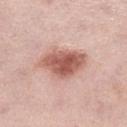Findings:
– TBP lesion metrics — a border-irregularity rating of about 2.5/10 and radial color variation of about 1.5
– lesion diameter — about 5.5 mm
– subject — female, aged around 65
– image — ~15 mm tile from a whole-body skin photo
– location — the leg